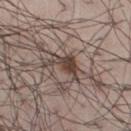The lesion was photographed on a routine skin check and not biopsied; there is no pathology result.
The tile uses white-light illumination.
Cropped from a total-body skin-imaging series; the visible field is about 15 mm.
The lesion is located on the left thigh.
An algorithmic analysis of the crop reported an eccentricity of roughly 0.85 and two-axis asymmetry of about 0.6. The analysis additionally found a color-variation rating of about 3/10 and radial color variation of about 1.
A male patient aged 63–67.
Approximately 4 mm at its widest.